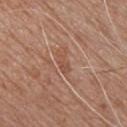notes — no biopsy performed (imaged during a skin exam) | patient — male, in their mid- to late 50s | diameter — about 3 mm | anatomic site — the chest | illumination — white-light illumination | image source — ~15 mm crop, total-body skin-cancer survey | image-analysis metrics — a lesion area of about 3 mm², an outline eccentricity of about 0.9 (0 = round, 1 = elongated), and a symmetry-axis asymmetry near 0.4; a classifier nevus-likeness of about 0/100.